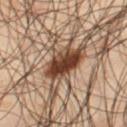biopsy_status: not biopsied; imaged during a skin examination
image:
  source: total-body photography crop
  field_of_view_mm: 15
site: leg
patient:
  sex: male
  age_approx: 50
automated_metrics:
  area_mm2_approx: 10.0
  eccentricity: 0.5
  shape_asymmetry: 0.15
  nevus_likeness_0_100: 100
  lesion_detection_confidence_0_100: 100
lesion_size:
  long_diameter_mm_approx: 4.0
lighting: cross-polarized